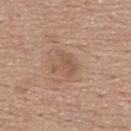Q: Was a biopsy performed?
A: imaged on a skin check; not biopsied
Q: What is the imaging modality?
A: 15 mm crop, total-body photography
Q: What is the lesion's diameter?
A: about 3 mm
Q: Patient demographics?
A: female, aged 58–62
Q: Where on the body is the lesion?
A: the upper back